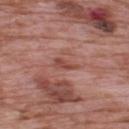<record>
  <biopsy_status>not biopsied; imaged during a skin examination</biopsy_status>
  <site>upper back</site>
  <automated_metrics>
    <border_irregularity_0_10>4.5</border_irregularity_0_10>
    <peripheral_color_asymmetry>0.5</peripheral_color_asymmetry>
  </automated_metrics>
  <lighting>white-light</lighting>
  <patient>
    <sex>male</sex>
    <age_approx>70</age_approx>
  </patient>
  <image>
    <source>total-body photography crop</source>
    <field_of_view_mm>15</field_of_view_mm>
  </image>
</record>